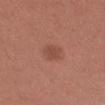Clinical impression: Imaged during a routine full-body skin examination; the lesion was not biopsied and no histopathology is available. Image and clinical context: A lesion tile, about 15 mm wide, cut from a 3D total-body photograph. Longest diameter approximately 2.5 mm. A female patient, in their mid- to late 20s. The lesion-visualizer software estimated a lesion–skin lightness drop of about 7 and a normalized border contrast of about 5.5. The analysis additionally found internal color variation of about 1.5 on a 0–10 scale. The lesion is on the left forearm. This is a white-light tile.